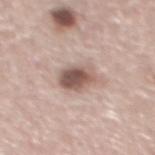<tbp_lesion>
<biopsy_status>not biopsied; imaged during a skin examination</biopsy_status>
<lighting>white-light</lighting>
<patient>
  <sex>male</sex>
  <age_approx>60</age_approx>
</patient>
<site>back</site>
<lesion_size>
  <long_diameter_mm_approx>4.5</long_diameter_mm_approx>
</lesion_size>
<image>
  <source>total-body photography crop</source>
  <field_of_view_mm>15</field_of_view_mm>
</image>
</tbp_lesion>